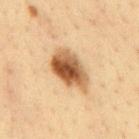{
  "biopsy_status": "not biopsied; imaged during a skin examination",
  "lighting": "cross-polarized",
  "patient": {
    "sex": "male",
    "age_approx": 35
  },
  "image": {
    "source": "total-body photography crop",
    "field_of_view_mm": 15
  },
  "lesion_size": {
    "long_diameter_mm_approx": 6.0
  },
  "automated_metrics": {
    "cielab_L": 53,
    "cielab_a": 20,
    "cielab_b": 36,
    "vs_skin_darker_L": 18.0,
    "vs_skin_contrast_norm": 11.5,
    "color_variation_0_10": 9.0
  },
  "site": "mid back"
}